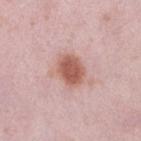Recorded during total-body skin imaging; not selected for excision or biopsy.